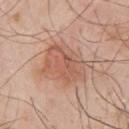Q: Is there a histopathology result?
A: total-body-photography surveillance lesion; no biopsy
Q: What kind of image is this?
A: ~15 mm tile from a whole-body skin photo
Q: Illumination type?
A: white-light
Q: What is the lesion's diameter?
A: ~6 mm (longest diameter)
Q: What did automated image analysis measure?
A: a footprint of about 20 mm² and a shape-asymmetry score of about 0.25 (0 = symmetric); a mean CIELAB color near L≈58 a*≈22 b*≈30 and a normalized border contrast of about 6.5; a peripheral color-asymmetry measure near 1.5
Q: Lesion location?
A: the chest
Q: Who is the patient?
A: male, aged approximately 55